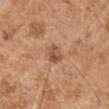Context: A male patient approximately 75 years of age. The lesion is located on the right upper arm. A roughly 15 mm field-of-view crop from a total-body skin photograph.